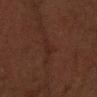Imaged during a routine full-body skin examination; the lesion was not biopsied and no histopathology is available. The patient is a female aged around 50. A 15 mm crop from a total-body photograph taken for skin-cancer surveillance. Captured under cross-polarized illumination. Located on the arm. The recorded lesion diameter is about 3 mm.